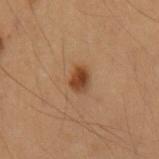The lesion was tiled from a total-body skin photograph and was not biopsied. Imaged with cross-polarized lighting. Automated image analysis of the tile measured a lesion area of about 4.5 mm², an outline eccentricity of about 0.6 (0 = round, 1 = elongated), and a symmetry-axis asymmetry near 0.2. The analysis additionally found a classifier nevus-likeness of about 95/100 and a detector confidence of about 100 out of 100 that the crop contains a lesion. The subject is a female approximately 40 years of age. From the right forearm. About 2.5 mm across. A 15 mm close-up tile from a total-body photography series done for melanoma screening.